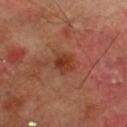Q: Was a biopsy performed?
A: catalogued during a skin exam; not biopsied
Q: What is the imaging modality?
A: 15 mm crop, total-body photography
Q: What are the patient's age and sex?
A: male, aged 68–72
Q: What is the anatomic site?
A: the right lower leg